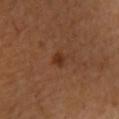The lesion was tiled from a total-body skin photograph and was not biopsied. The tile uses cross-polarized illumination. A 15 mm close-up tile from a total-body photography series done for melanoma screening. On the arm. The lesion's longest dimension is about 2 mm. A female patient, aged 28–32.